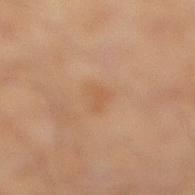  biopsy_status: not biopsied; imaged during a skin examination
  patient:
    sex: male
    age_approx: 60
  image:
    source: total-body photography crop
    field_of_view_mm: 15
  site: leg
  lesion_size:
    long_diameter_mm_approx: 2.5
  lighting: cross-polarized